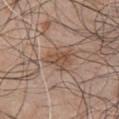Q: Was a biopsy performed?
A: total-body-photography surveillance lesion; no biopsy